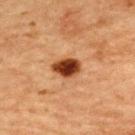This lesion was catalogued during total-body skin photography and was not selected for biopsy.
The lesion is located on the upper back.
Measured at roughly 3.5 mm in maximum diameter.
A 15 mm close-up tile from a total-body photography series done for melanoma screening.
A female patient in their 70s.